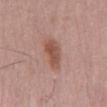The lesion was photographed on a routine skin check and not biopsied; there is no pathology result. A region of skin cropped from a whole-body photographic capture, roughly 15 mm wide. A male patient, aged 53 to 57. This is a white-light tile.